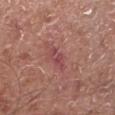This lesion was catalogued during total-body skin photography and was not selected for biopsy. The recorded lesion diameter is about 2.5 mm. A male subject about 55 years old. On the leg. A 15 mm crop from a total-body photograph taken for skin-cancer surveillance.